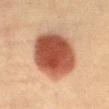  biopsy_status: not biopsied; imaged during a skin examination
  automated_metrics:
    area_mm2_approx: 32.0
    eccentricity: 0.5
    shape_asymmetry: 0.1
    color_variation_0_10: 6.0
    peripheral_color_asymmetry: 1.5
    nevus_likeness_0_100: 100
    lesion_detection_confidence_0_100: 100
  image:
    source: total-body photography crop
    field_of_view_mm: 15
  patient:
    sex: female
    age_approx: 40
  lesion_size:
    long_diameter_mm_approx: 6.5
  site: abdomen
  lighting: cross-polarized